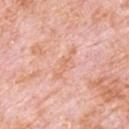Part of a total-body skin-imaging series; this lesion was reviewed on a skin check and was not flagged for biopsy.
A 15 mm crop from a total-body photograph taken for skin-cancer surveillance.
Imaged with white-light lighting.
An algorithmic analysis of the crop reported a footprint of about 6 mm² and a shape-asymmetry score of about 0.4 (0 = symmetric). And it measured a lesion color around L≈69 a*≈24 b*≈33 in CIELAB, a lesion–skin lightness drop of about 6, and a normalized lesion–skin contrast near 5. The analysis additionally found a border-irregularity index near 5.5/10, a color-variation rating of about 2/10, and peripheral color asymmetry of about 0.5.
The subject is a male aged 78–82.
From the upper back.